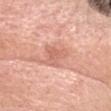Captured during whole-body skin photography for melanoma surveillance; the lesion was not biopsied.
Captured under white-light illumination.
The subject is a female aged 53–57.
Automated tile analysis of the lesion measured a lesion area of about 6.5 mm² and two-axis asymmetry of about 0.5. The analysis additionally found a mean CIELAB color near L≈64 a*≈26 b*≈30, about 9 CIELAB-L* units darker than the surrounding skin, and a normalized border contrast of about 5.5. The software also gave border irregularity of about 5 on a 0–10 scale and internal color variation of about 3 on a 0–10 scale.
Measured at roughly 4 mm in maximum diameter.
A 15 mm close-up extracted from a 3D total-body photography capture.
Located on the head or neck.